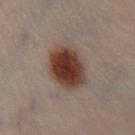{"biopsy_status": "not biopsied; imaged during a skin examination", "lesion_size": {"long_diameter_mm_approx": 5.0}, "lighting": "cross-polarized", "patient": {"sex": "male", "age_approx": 50}, "site": "left leg", "image": {"source": "total-body photography crop", "field_of_view_mm": 15}}